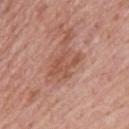Case summary:
• biopsy status: catalogued during a skin exam; not biopsied
• diameter: ≈4 mm
• anatomic site: the upper back
• tile lighting: white-light illumination
• automated metrics: an automated nevus-likeness rating near 0 out of 100 and a lesion-detection confidence of about 100/100
• patient: male, aged around 75
• image: total-body-photography crop, ~15 mm field of view